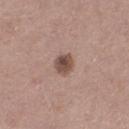Findings:
- follow-up · total-body-photography surveillance lesion; no biopsy
- anatomic site · the leg
- image-analysis metrics · a classifier nevus-likeness of about 90/100
- patient · female, about 40 years old
- image source · ~15 mm tile from a whole-body skin photo
- illumination · white-light illumination